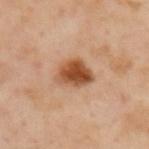Notes:
• anatomic site: the upper back
• image source: ~15 mm tile from a whole-body skin photo
• subject: male, aged 53–57